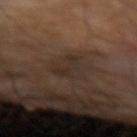Part of a total-body skin-imaging series; this lesion was reviewed on a skin check and was not flagged for biopsy. A male subject about 70 years old. From the left forearm. The tile uses cross-polarized illumination. A 15 mm close-up tile from a total-body photography series done for melanoma screening.